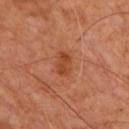Captured during whole-body skin photography for melanoma surveillance; the lesion was not biopsied.
The subject is a male in their mid- to late 60s.
Cropped from a whole-body photographic skin survey; the tile spans about 15 mm.
The lesion's longest dimension is about 3 mm.
Captured under cross-polarized illumination.
Automated image analysis of the tile measured a lesion area of about 4.5 mm², an eccentricity of roughly 0.8, and a symmetry-axis asymmetry near 0.2. It also reported an average lesion color of about L≈44 a*≈27 b*≈36 (CIELAB), a lesion–skin lightness drop of about 8, and a lesion-to-skin contrast of about 7 (normalized; higher = more distinct). And it measured a border-irregularity index near 2.5/10, a color-variation rating of about 2/10, and radial color variation of about 0.5.